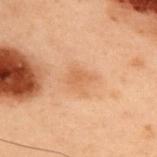Assessment: Imaged during a routine full-body skin examination; the lesion was not biopsied and no histopathology is available. Clinical summary: A close-up tile cropped from a whole-body skin photograph, about 15 mm across. The subject is a male in their mid-50s. The lesion is on the upper back.